About 9.5 mm across; imaged with white-light lighting; a female subject, approximately 65 years of age; the lesion is located on the right lower leg; this image is a 15 mm lesion crop taken from a total-body photograph.
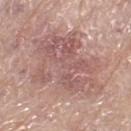histopathologic diagnosis: an actinic keratosis (borderline).